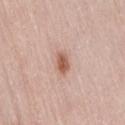Q: Was a biopsy performed?
A: no biopsy performed (imaged during a skin exam)
Q: What lighting was used for the tile?
A: white-light
Q: How large is the lesion?
A: ~2.5 mm (longest diameter)
Q: Where on the body is the lesion?
A: the back
Q: What kind of image is this?
A: total-body-photography crop, ~15 mm field of view
Q: Automated lesion metrics?
A: a lesion area of about 4 mm² and two-axis asymmetry of about 0.25; an average lesion color of about L≈58 a*≈22 b*≈29 (CIELAB), roughly 12 lightness units darker than nearby skin, and a normalized border contrast of about 8.5
Q: Patient demographics?
A: female, about 65 years old